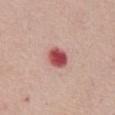Measured at roughly 3 mm in maximum diameter. A female patient aged around 65. Cropped from a total-body skin-imaging series; the visible field is about 15 mm. Captured under white-light illumination. On the chest.